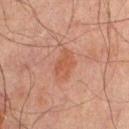This lesion was catalogued during total-body skin photography and was not selected for biopsy. Automated image analysis of the tile measured a mean CIELAB color near L≈42 a*≈22 b*≈27, roughly 6 lightness units darker than nearby skin, and a normalized lesion–skin contrast near 6. Longest diameter approximately 3 mm. The tile uses cross-polarized illumination. A 15 mm close-up tile from a total-body photography series done for melanoma screening. A male patient in their mid-60s. The lesion is located on the upper back.